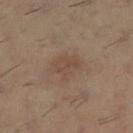notes: no biopsy performed (imaged during a skin exam) | subject: male, about 30 years old | image source: 15 mm crop, total-body photography | anatomic site: the left lower leg | image-analysis metrics: a lesion area of about 8 mm² and a symmetry-axis asymmetry near 0.25; border irregularity of about 3 on a 0–10 scale, internal color variation of about 1.5 on a 0–10 scale, and peripheral color asymmetry of about 0.5 | illumination: cross-polarized | size: ≈4 mm.